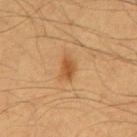Clinical impression: Part of a total-body skin-imaging series; this lesion was reviewed on a skin check and was not flagged for biopsy. Clinical summary: On the abdomen. This image is a 15 mm lesion crop taken from a total-body photograph. Captured under cross-polarized illumination. The patient is a male aged 58 to 62. About 2.5 mm across.